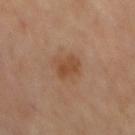biopsy_status: not biopsied; imaged during a skin examination
lesion_size:
  long_diameter_mm_approx: 3.5
site: mid back
lighting: cross-polarized
image:
  source: total-body photography crop
  field_of_view_mm: 15
automated_metrics:
  area_mm2_approx: 7.0
  eccentricity: 0.7
  shape_asymmetry: 0.25
  border_irregularity_0_10: 2.5
  color_variation_0_10: 2.5
  peripheral_color_asymmetry: 1.0